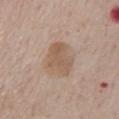  biopsy_status: not biopsied; imaged during a skin examination
  lighting: white-light
  lesion_size:
    long_diameter_mm_approx: 4.0
  patient:
    sex: male
    age_approx: 75
  image:
    source: total-body photography crop
    field_of_view_mm: 15
  site: mid back